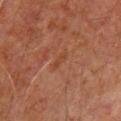  biopsy_status: not biopsied; imaged during a skin examination
  lesion_size:
    long_diameter_mm_approx: 2.5
  site: chest
  image:
    source: total-body photography crop
    field_of_view_mm: 15
  patient:
    sex: male
    age_approx: 60